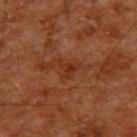workup: catalogued during a skin exam; not biopsied
imaging modality: 15 mm crop, total-body photography
lighting: cross-polarized
anatomic site: the upper back
patient: male, aged 58–62
image-analysis metrics: a lesion area of about 3.5 mm² and an outline eccentricity of about 0.55 (0 = round, 1 = elongated); border irregularity of about 6 on a 0–10 scale, a color-variation rating of about 1/10, and a peripheral color-asymmetry measure near 0; a nevus-likeness score of about 0/100 and lesion-presence confidence of about 100/100
lesion diameter: ~3 mm (longest diameter)